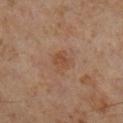| key | value |
|---|---|
| biopsy status | imaged on a skin check; not biopsied |
| subject | male, roughly 60 years of age |
| tile lighting | cross-polarized illumination |
| diameter | ~2.5 mm (longest diameter) |
| site | the right lower leg |
| acquisition | total-body-photography crop, ~15 mm field of view |